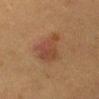Clinical impression: Captured during whole-body skin photography for melanoma surveillance; the lesion was not biopsied. Clinical summary: A female patient aged around 50. The lesion-visualizer software estimated a footprint of about 12 mm², an eccentricity of roughly 0.75, and two-axis asymmetry of about 0.4. The software also gave an automated nevus-likeness rating near 80 out of 100. Cropped from a whole-body photographic skin survey; the tile spans about 15 mm. On the front of the torso. Captured under cross-polarized illumination. Longest diameter approximately 5 mm.